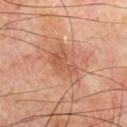Q: Automated lesion metrics?
A: an area of roughly 10 mm², a shape eccentricity near 0.85, and a symmetry-axis asymmetry near 0.35; a border-irregularity index near 4/10, internal color variation of about 4.5 on a 0–10 scale, and a peripheral color-asymmetry measure near 1.5
Q: What is the anatomic site?
A: the chest
Q: What kind of image is this?
A: total-body-photography crop, ~15 mm field of view
Q: Illumination type?
A: cross-polarized illumination
Q: Who is the patient?
A: male, approximately 70 years of age